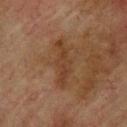follow-up: total-body-photography surveillance lesion; no biopsy | body site: the upper back | image source: ~15 mm tile from a whole-body skin photo | lighting: cross-polarized illumination | patient: male, approximately 75 years of age.